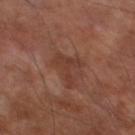follow-up = imaged on a skin check; not biopsied
site = the left lower leg
lesion diameter = about 4.5 mm
patient = male, about 70 years old
automated lesion analysis = an eccentricity of roughly 0.75; a lesion color around L≈36 a*≈22 b*≈26 in CIELAB, a lesion–skin lightness drop of about 6, and a normalized border contrast of about 6; a color-variation rating of about 1/10 and radial color variation of about 0.5
illumination = cross-polarized illumination
imaging modality = total-body-photography crop, ~15 mm field of view